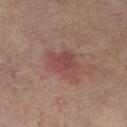This lesion was catalogued during total-body skin photography and was not selected for biopsy.
An algorithmic analysis of the crop reported internal color variation of about 2.5 on a 0–10 scale.
A 15 mm close-up tile from a total-body photography series done for melanoma screening.
From the left lower leg.
A female patient, aged around 50.